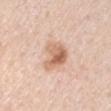Imaged during a routine full-body skin examination; the lesion was not biopsied and no histopathology is available.
The subject is a male aged 38 to 42.
Longest diameter approximately 4 mm.
A 15 mm close-up tile from a total-body photography series done for melanoma screening.
Automated image analysis of the tile measured a lesion color around L≈65 a*≈21 b*≈32 in CIELAB, roughly 13 lightness units darker than nearby skin, and a normalized lesion–skin contrast near 8. And it measured a nevus-likeness score of about 70/100 and a lesion-detection confidence of about 100/100.
On the right upper arm.